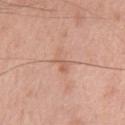No biopsy was performed on this lesion — it was imaged during a full skin examination and was not determined to be concerning. A male patient, roughly 50 years of age. The lesion is located on the chest. Cropped from a total-body skin-imaging series; the visible field is about 15 mm.